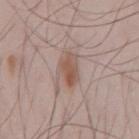This lesion was catalogued during total-body skin photography and was not selected for biopsy. A male subject, about 45 years old. Captured under white-light illumination. On the front of the torso. About 4 mm across. A close-up tile cropped from a whole-body skin photograph, about 15 mm across. An algorithmic analysis of the crop reported a footprint of about 7 mm², a shape eccentricity near 0.8, and two-axis asymmetry of about 0.2. The software also gave a border-irregularity rating of about 2.5/10 and a within-lesion color-variation index near 4/10.